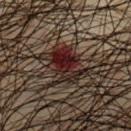Captured during whole-body skin photography for melanoma surveillance; the lesion was not biopsied.
The total-body-photography lesion software estimated a footprint of about 10 mm², an outline eccentricity of about 0.8 (0 = round, 1 = elongated), and a symmetry-axis asymmetry near 0.25. The analysis additionally found a mean CIELAB color near L≈17 a*≈17 b*≈16, a lesion–skin lightness drop of about 11, and a normalized border contrast of about 13.5. It also reported a nevus-likeness score of about 0/100 and lesion-presence confidence of about 100/100.
On the chest.
The recorded lesion diameter is about 4.5 mm.
Captured under cross-polarized illumination.
Cropped from a whole-body photographic skin survey; the tile spans about 15 mm.
A male patient in their mid- to late 60s.